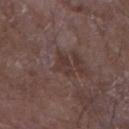– follow-up — no biopsy performed (imaged during a skin exam)
– tile lighting — white-light illumination
– site — the left forearm
– automated metrics — a footprint of about 4.5 mm², a shape eccentricity near 0.75, and a symmetry-axis asymmetry near 0.4; a lesion–skin lightness drop of about 6 and a lesion-to-skin contrast of about 6 (normalized; higher = more distinct); a within-lesion color-variation index near 2.5/10 and a peripheral color-asymmetry measure near 1; a nevus-likeness score of about 0/100 and lesion-presence confidence of about 60/100
– subject — male, approximately 65 years of age
– lesion diameter — ≈3 mm
– image — ~15 mm tile from a whole-body skin photo